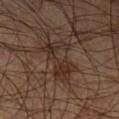Case summary:
– site · the left lower leg
– image · 15 mm crop, total-body photography
– subject · male, about 60 years old
– tile lighting · cross-polarized illumination